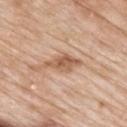Findings:
* workup · total-body-photography surveillance lesion; no biopsy
* subject · male, about 85 years old or older
* body site · the upper back
* automated lesion analysis · a lesion area of about 7 mm², a shape eccentricity near 0.9, and two-axis asymmetry of about 0.25; a detector confidence of about 100 out of 100 that the crop contains a lesion
* image · total-body-photography crop, ~15 mm field of view
* lighting · white-light illumination